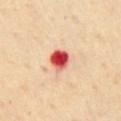Case summary:
- workup: imaged on a skin check; not biopsied
- body site: the chest
- image: ~15 mm crop, total-body skin-cancer survey
- patient: male, in their mid-50s